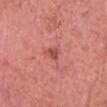Assessment:
No biopsy was performed on this lesion — it was imaged during a full skin examination and was not determined to be concerning.
Context:
Captured under white-light illumination. A roughly 15 mm field-of-view crop from a total-body skin photograph. About 2.5 mm across. Automated tile analysis of the lesion measured a lesion–skin lightness drop of about 10 and a normalized lesion–skin contrast near 7. The analysis additionally found a border-irregularity index near 3.5/10, internal color variation of about 1.5 on a 0–10 scale, and radial color variation of about 0.5. The lesion is on the head or neck. The subject is a male aged 78 to 82.